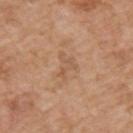Q: Is there a histopathology result?
A: no biopsy performed (imaged during a skin exam)
Q: What is the anatomic site?
A: the upper back
Q: How was this image acquired?
A: ~15 mm tile from a whole-body skin photo
Q: How large is the lesion?
A: ~3 mm (longest diameter)
Q: Patient demographics?
A: male, in their 60s
Q: What lighting was used for the tile?
A: white-light illumination